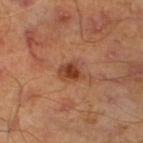Clinical impression:
The lesion was tiled from a total-body skin photograph and was not biopsied.
Image and clinical context:
A region of skin cropped from a whole-body photographic capture, roughly 15 mm wide. The lesion is located on the left lower leg. Automated image analysis of the tile measured a symmetry-axis asymmetry near 0.25. It also reported a lesion color around L≈42 a*≈25 b*≈33 in CIELAB, roughly 11 lightness units darker than nearby skin, and a normalized lesion–skin contrast near 8.5. The software also gave a border-irregularity rating of about 2.5/10, a within-lesion color-variation index near 4/10, and radial color variation of about 1. Approximately 3 mm at its widest. Captured under cross-polarized illumination. A male subject aged around 45.